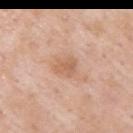The lesion was photographed on a routine skin check and not biopsied; there is no pathology result. A region of skin cropped from a whole-body photographic capture, roughly 15 mm wide. Captured under white-light illumination. Measured at roughly 2.5 mm in maximum diameter. From the right upper arm. A male subject roughly 55 years of age.